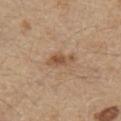Imaged during a routine full-body skin examination; the lesion was not biopsied and no histopathology is available. The lesion is located on the chest. A male patient roughly 70 years of age. A roughly 15 mm field-of-view crop from a total-body skin photograph.